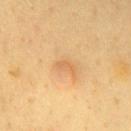{"biopsy_status": "not biopsied; imaged during a skin examination", "site": "chest", "image": {"source": "total-body photography crop", "field_of_view_mm": 15}, "patient": {"sex": "male", "age_approx": 65}, "lighting": "cross-polarized"}